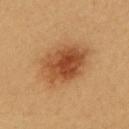Automated image analysis of the tile measured a detector confidence of about 100 out of 100 that the crop contains a lesion. From the upper back. A close-up tile cropped from a whole-body skin photograph, about 15 mm across. A female subject aged 23 to 27.